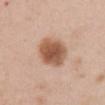biopsy status = catalogued during a skin exam; not biopsied | lesion size = ≈5 mm | patient = female, about 50 years old | imaging modality = ~15 mm tile from a whole-body skin photo | illumination = white-light illumination | location = the mid back.